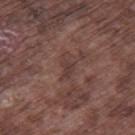The lesion is on the right thigh. Automated image analysis of the tile measured an area of roughly 4 mm². And it measured a lesion-to-skin contrast of about 6 (normalized; higher = more distinct). The software also gave border irregularity of about 4 on a 0–10 scale, internal color variation of about 1 on a 0–10 scale, and radial color variation of about 0.5. Approximately 3 mm at its widest. A male subject, roughly 75 years of age. Imaged with white-light lighting. A close-up tile cropped from a whole-body skin photograph, about 15 mm across.